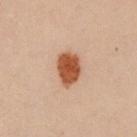Q: Is there a histopathology result?
A: no biopsy performed (imaged during a skin exam)
Q: Patient demographics?
A: female, aged 28–32
Q: What did automated image analysis measure?
A: an average lesion color of about L≈41 a*≈22 b*≈29 (CIELAB) and roughly 14 lightness units darker than nearby skin; a classifier nevus-likeness of about 100/100
Q: Illumination type?
A: cross-polarized illumination
Q: What kind of image is this?
A: total-body-photography crop, ~15 mm field of view
Q: What is the anatomic site?
A: the left upper arm
Q: How large is the lesion?
A: ~4 mm (longest diameter)